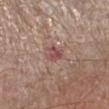Notes:
- follow-up — no biopsy performed (imaged during a skin exam)
- patient — male, in their mid-50s
- lesion diameter — about 2.5 mm
- location — the leg
- acquisition — ~15 mm tile from a whole-body skin photo